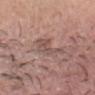Q: Is there a histopathology result?
A: no biopsy performed (imaged during a skin exam)
Q: What is the lesion's diameter?
A: ~4.5 mm (longest diameter)
Q: What lighting was used for the tile?
A: white-light
Q: Who is the patient?
A: male, aged 28 to 32
Q: Lesion location?
A: the head or neck
Q: How was this image acquired?
A: 15 mm crop, total-body photography
Q: Automated lesion metrics?
A: an area of roughly 6.5 mm² and a shape eccentricity near 0.9; a lesion–skin lightness drop of about 8 and a lesion-to-skin contrast of about 5.5 (normalized; higher = more distinct); a classifier nevus-likeness of about 0/100 and a detector confidence of about 80 out of 100 that the crop contains a lesion